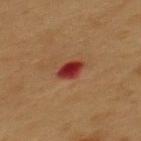This lesion was catalogued during total-body skin photography and was not selected for biopsy.
The lesion's longest dimension is about 2.5 mm.
Cropped from a whole-body photographic skin survey; the tile spans about 15 mm.
This is a cross-polarized tile.
The lesion is located on the mid back.
A male subject approximately 55 years of age.